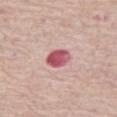About 3 mm across. Automated tile analysis of the lesion measured a lesion area of about 6 mm², an eccentricity of roughly 0.45, and two-axis asymmetry of about 0.15. The analysis additionally found a lesion color around L≈54 a*≈34 b*≈19 in CIELAB and roughly 17 lightness units darker than nearby skin. The analysis additionally found a border-irregularity rating of about 1.5/10 and radial color variation of about 1.5. It also reported a nevus-likeness score of about 0/100. From the right thigh. A region of skin cropped from a whole-body photographic capture, roughly 15 mm wide. This is a white-light tile. A female patient approximately 70 years of age.